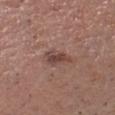{"biopsy_status": "not biopsied; imaged during a skin examination", "image": {"source": "total-body photography crop", "field_of_view_mm": 15}, "automated_metrics": {"area_mm2_approx": 4.5, "eccentricity": 0.8, "shape_asymmetry": 0.4, "vs_skin_contrast_norm": 7.5}, "patient": {"sex": "male", "age_approx": 55}, "site": "head or neck", "lesion_size": {"long_diameter_mm_approx": 3.0}}